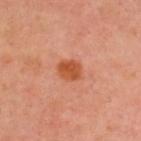Recorded during total-body skin imaging; not selected for excision or biopsy. The lesion is located on the upper back. A male subject in their 50s. The tile uses cross-polarized illumination. The lesion-visualizer software estimated a classifier nevus-likeness of about 95/100 and lesion-presence confidence of about 100/100. A roughly 15 mm field-of-view crop from a total-body skin photograph. About 2.5 mm across.